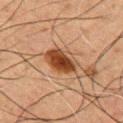Recorded during total-body skin imaging; not selected for excision or biopsy. From the chest. Captured under cross-polarized illumination. Automated image analysis of the tile measured a nevus-likeness score of about 95/100 and lesion-presence confidence of about 100/100. A 15 mm close-up extracted from a 3D total-body photography capture. Approximately 4 mm at its widest. The patient is a male aged 48 to 52.